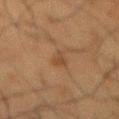The lesion was photographed on a routine skin check and not biopsied; there is no pathology result.
The lesion is on the back.
A roughly 15 mm field-of-view crop from a total-body skin photograph.
A male subject, about 60 years old.